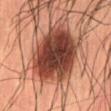{"biopsy_status": "not biopsied; imaged during a skin examination", "site": "back", "patient": {"sex": "male", "age_approx": 55}, "image": {"source": "total-body photography crop", "field_of_view_mm": 15}, "automated_metrics": {"area_mm2_approx": 34.0, "shape_asymmetry": 0.15}, "lesion_size": {"long_diameter_mm_approx": 8.5}, "lighting": "cross-polarized"}